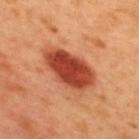follow-up=no biopsy performed (imaged during a skin exam) | subject=male, about 50 years old | body site=the upper back | imaging modality=total-body-photography crop, ~15 mm field of view.